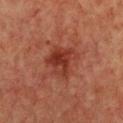Imaged during a routine full-body skin examination; the lesion was not biopsied and no histopathology is available. Located on the chest. A 15 mm close-up extracted from a 3D total-body photography capture. Longest diameter approximately 4 mm. A female patient, aged 53–57.